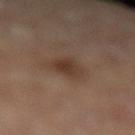Assessment: Imaged during a routine full-body skin examination; the lesion was not biopsied and no histopathology is available. Acquisition and patient details: A lesion tile, about 15 mm wide, cut from a 3D total-body photograph. A female patient, aged approximately 60. An algorithmic analysis of the crop reported a border-irregularity rating of about 3/10, a within-lesion color-variation index near 2.5/10, and a peripheral color-asymmetry measure near 0.5. The recorded lesion diameter is about 3.5 mm. The lesion is on the left lower leg.